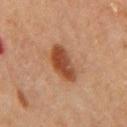The lesion was tiled from a total-body skin photograph and was not biopsied.
Approximately 4.5 mm at its widest.
A 15 mm crop from a total-body photograph taken for skin-cancer surveillance.
The lesion is on the back.
A male patient in their mid-60s.
The tile uses cross-polarized illumination.